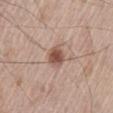Q: Is there a histopathology result?
A: no biopsy performed (imaged during a skin exam)
Q: Lesion location?
A: the abdomen
Q: Patient demographics?
A: male, aged 68–72
Q: How was this image acquired?
A: 15 mm crop, total-body photography